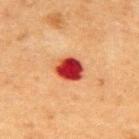– follow-up · no biopsy performed (imaged during a skin exam)
– subject · male, approximately 50 years of age
– diameter · ~3.5 mm (longest diameter)
– location · the upper back
– TBP lesion metrics · a lesion area of about 8 mm², a shape eccentricity near 0.55, and two-axis asymmetry of about 0.15; a lesion color around L≈37 a*≈37 b*≈32 in CIELAB and about 20 CIELAB-L* units darker than the surrounding skin
– tile lighting · cross-polarized illumination
– imaging modality · ~15 mm tile from a whole-body skin photo